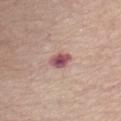<tbp_lesion>
  <biopsy_status>not biopsied; imaged during a skin examination</biopsy_status>
  <image>
    <source>total-body photography crop</source>
    <field_of_view_mm>15</field_of_view_mm>
  </image>
  <automated_metrics>
    <cielab_L>52</cielab_L>
    <cielab_a>26</cielab_a>
    <cielab_b>18</cielab_b>
    <vs_skin_darker_L>15.0</vs_skin_darker_L>
    <vs_skin_contrast_norm>11.0</vs_skin_contrast_norm>
    <border_irregularity_0_10>2.0</border_irregularity_0_10>
    <color_variation_0_10>4.5</color_variation_0_10>
    <peripheral_color_asymmetry>1.5</peripheral_color_asymmetry>
  </automated_metrics>
  <lighting>white-light</lighting>
  <patient>
    <sex>female</sex>
    <age_approx>70</age_approx>
  </patient>
  <site>chest</site>
  <lesion_size>
    <long_diameter_mm_approx>2.5</long_diameter_mm_approx>
  </lesion_size>
</tbp_lesion>